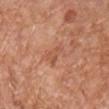The lesion was photographed on a routine skin check and not biopsied; there is no pathology result. A 15 mm close-up tile from a total-body photography series done for melanoma screening. The lesion is located on the chest. A male subject approximately 65 years of age. The lesion's longest dimension is about 3 mm.